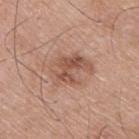Part of a total-body skin-imaging series; this lesion was reviewed on a skin check and was not flagged for biopsy.
Approximately 5 mm at its widest.
The lesion is located on the upper back.
Automated tile analysis of the lesion measured border irregularity of about 5.5 on a 0–10 scale, a color-variation rating of about 6/10, and a peripheral color-asymmetry measure near 2. And it measured a classifier nevus-likeness of about 5/100 and lesion-presence confidence of about 100/100.
The subject is a male aged around 75.
A 15 mm crop from a total-body photograph taken for skin-cancer surveillance.